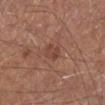Part of a total-body skin-imaging series; this lesion was reviewed on a skin check and was not flagged for biopsy. A lesion tile, about 15 mm wide, cut from a 3D total-body photograph. A male subject, aged around 70. From the left lower leg.